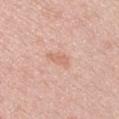{"biopsy_status": "not biopsied; imaged during a skin examination", "patient": {"sex": "male", "age_approx": 45}, "lighting": "white-light", "site": "left upper arm", "image": {"source": "total-body photography crop", "field_of_view_mm": 15}}